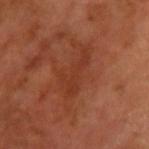Q: Was a biopsy performed?
A: catalogued during a skin exam; not biopsied
Q: Patient demographics?
A: male, aged 63 to 67
Q: How was this image acquired?
A: ~15 mm crop, total-body skin-cancer survey